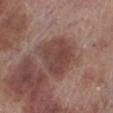| feature | finding |
|---|---|
| follow-up | total-body-photography surveillance lesion; no biopsy |
| patient | male, in their 70s |
| image | total-body-photography crop, ~15 mm field of view |
| TBP lesion metrics | an area of roughly 18 mm², an outline eccentricity of about 0.5 (0 = round, 1 = elongated), and a shape-asymmetry score of about 0.25 (0 = symmetric) |
| body site | the left lower leg |
| lighting | white-light illumination |
| diameter | ~5.5 mm (longest diameter) |